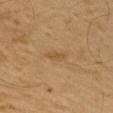Captured under cross-polarized illumination.
A male subject in their 60s.
Located on the right upper arm.
A roughly 15 mm field-of-view crop from a total-body skin photograph.